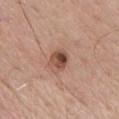<case>
<biopsy_status>not biopsied; imaged during a skin examination</biopsy_status>
<lesion_size>
  <long_diameter_mm_approx>2.5</long_diameter_mm_approx>
</lesion_size>
<patient>
  <sex>male</sex>
  <age_approx>65</age_approx>
</patient>
<image>
  <source>total-body photography crop</source>
  <field_of_view_mm>15</field_of_view_mm>
</image>
<site>chest</site>
</case>